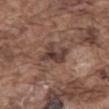Notes:
- biopsy status — no biopsy performed (imaged during a skin exam)
- site — the front of the torso
- subject — male, in their mid- to late 70s
- illumination — white-light illumination
- lesion size — ≈4 mm
- image — 15 mm crop, total-body photography